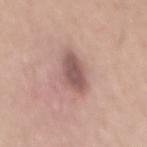Q: What is the lesion's diameter?
A: about 5.5 mm
Q: Lesion location?
A: the mid back
Q: What are the patient's age and sex?
A: male, approximately 30 years of age
Q: What is the imaging modality?
A: 15 mm crop, total-body photography
Q: Automated lesion metrics?
A: a footprint of about 10 mm² and a shape eccentricity near 0.85; a mean CIELAB color near L≈55 a*≈20 b*≈22, a lesion–skin lightness drop of about 13, and a normalized border contrast of about 8.5; a border-irregularity rating of about 3/10 and peripheral color asymmetry of about 1
Q: How was the tile lit?
A: white-light